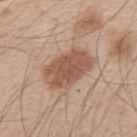| field | value |
|---|---|
| notes | total-body-photography surveillance lesion; no biopsy |
| location | the back |
| illumination | white-light illumination |
| image | 15 mm crop, total-body photography |
| image-analysis metrics | a border-irregularity index near 2/10, internal color variation of about 3.5 on a 0–10 scale, and radial color variation of about 1 |
| patient | male, aged 48 to 52 |
| size | ≈6 mm |